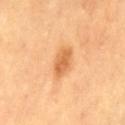No biopsy was performed on this lesion — it was imaged during a full skin examination and was not determined to be concerning. A 15 mm crop from a total-body photograph taken for skin-cancer surveillance. A female subject aged 68–72. Automated image analysis of the tile measured border irregularity of about 2 on a 0–10 scale, a color-variation rating of about 2.5/10, and peripheral color asymmetry of about 1. And it measured a nevus-likeness score of about 85/100. Approximately 3 mm at its widest. Located on the leg. This is a cross-polarized tile.